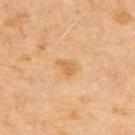Assessment:
Recorded during total-body skin imaging; not selected for excision or biopsy.
Image and clinical context:
A close-up tile cropped from a whole-body skin photograph, about 15 mm across. Approximately 2.5 mm at its widest. The lesion is on the upper back. A male subject, in their 70s. This is a cross-polarized tile.